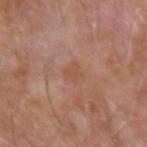Captured during whole-body skin photography for melanoma surveillance; the lesion was not biopsied.
Cropped from a total-body skin-imaging series; the visible field is about 15 mm.
A male subject, aged 73 to 77.
Located on the right forearm.
Approximately 2.5 mm at its widest.
Automated tile analysis of the lesion measured a border-irregularity rating of about 3/10, internal color variation of about 1 on a 0–10 scale, and radial color variation of about 0.5.